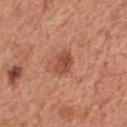biopsy status: catalogued during a skin exam; not biopsied
image: ~15 mm crop, total-body skin-cancer survey
location: the chest
lesion size: ≈3 mm
patient: male, aged 63–67
illumination: white-light